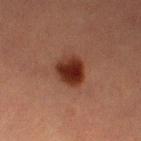The lesion was tiled from a total-body skin photograph and was not biopsied.
A female subject aged 53 to 57.
The lesion is located on the left thigh.
A lesion tile, about 15 mm wide, cut from a 3D total-body photograph.